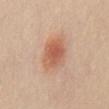Captured during whole-body skin photography for melanoma surveillance; the lesion was not biopsied. The lesion's longest dimension is about 4.5 mm. A female subject, roughly 20 years of age. A lesion tile, about 15 mm wide, cut from a 3D total-body photograph. The lesion is located on the chest.